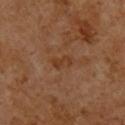notes: total-body-photography surveillance lesion; no biopsy | imaging modality: total-body-photography crop, ~15 mm field of view | lesion diameter: ≈3 mm | TBP lesion metrics: an average lesion color of about L≈36 a*≈20 b*≈32 (CIELAB), about 6 CIELAB-L* units darker than the surrounding skin, and a lesion-to-skin contrast of about 6.5 (normalized; higher = more distinct) | subject: male, in their 60s | body site: the upper back.